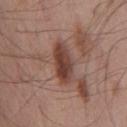  biopsy_status: not biopsied; imaged during a skin examination
  patient:
    sex: male
    age_approx: 55
  lighting: white-light
  image:
    source: total-body photography crop
    field_of_view_mm: 15
  site: chest